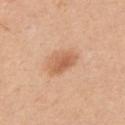follow-up: total-body-photography surveillance lesion; no biopsy
lesion diameter: about 4 mm
subject: male, about 50 years old
tile lighting: white-light illumination
acquisition: 15 mm crop, total-body photography
location: the chest
automated metrics: a lesion color around L≈61 a*≈23 b*≈35 in CIELAB and a normalized border contrast of about 7; a border-irregularity index near 2/10, a color-variation rating of about 3.5/10, and radial color variation of about 1; lesion-presence confidence of about 100/100